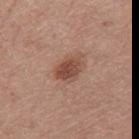Q: Was a biopsy performed?
A: total-body-photography surveillance lesion; no biopsy